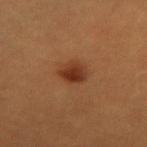Clinical impression:
The lesion was photographed on a routine skin check and not biopsied; there is no pathology result.
Acquisition and patient details:
On the abdomen. The lesion's longest dimension is about 3 mm. Imaged with cross-polarized lighting. Cropped from a whole-body photographic skin survey; the tile spans about 15 mm. An algorithmic analysis of the crop reported a mean CIELAB color near L≈32 a*≈23 b*≈31 and a normalized border contrast of about 9. The software also gave a peripheral color-asymmetry measure near 1. It also reported a classifier nevus-likeness of about 100/100 and a lesion-detection confidence of about 100/100. A female patient, aged around 30.